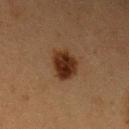The lesion was photographed on a routine skin check and not biopsied; there is no pathology result. Cropped from a total-body skin-imaging series; the visible field is about 15 mm. Automated tile analysis of the lesion measured a lesion area of about 9 mm² and an outline eccentricity of about 0.6 (0 = round, 1 = elongated). And it measured a normalized lesion–skin contrast near 12.5. A female patient aged 38–42. On the left upper arm.